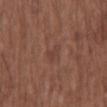biopsy status: imaged on a skin check; not biopsied
patient: female, aged 73 to 77
diameter: about 2.5 mm
tile lighting: white-light illumination
image source: ~15 mm crop, total-body skin-cancer survey
automated lesion analysis: an average lesion color of about L≈40 a*≈20 b*≈25 (CIELAB), about 5 CIELAB-L* units darker than the surrounding skin, and a normalized lesion–skin contrast near 4.5
anatomic site: the chest